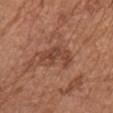No biopsy was performed on this lesion — it was imaged during a full skin examination and was not determined to be concerning. A female subject, about 75 years old. The recorded lesion diameter is about 4.5 mm. The tile uses white-light illumination. Cropped from a total-body skin-imaging series; the visible field is about 15 mm. On the chest.